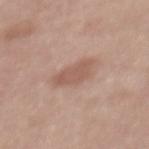Part of a total-body skin-imaging series; this lesion was reviewed on a skin check and was not flagged for biopsy.